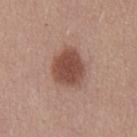workup: total-body-photography surveillance lesion; no biopsy
location: the front of the torso
acquisition: total-body-photography crop, ~15 mm field of view
subject: male, approximately 25 years of age